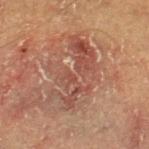Imaged during a routine full-body skin examination; the lesion was not biopsied and no histopathology is available. This is a cross-polarized tile. The lesion is located on the right thigh. About 8 mm across. An algorithmic analysis of the crop reported a mean CIELAB color near L≈44 a*≈21 b*≈26, about 8 CIELAB-L* units darker than the surrounding skin, and a normalized lesion–skin contrast near 6.5. And it measured a border-irregularity rating of about 8.5/10, internal color variation of about 5.5 on a 0–10 scale, and a peripheral color-asymmetry measure near 2. The analysis additionally found a nevus-likeness score of about 0/100. Cropped from a whole-body photographic skin survey; the tile spans about 15 mm. A male subject aged around 65.